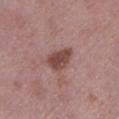This lesion was catalogued during total-body skin photography and was not selected for biopsy. A region of skin cropped from a whole-body photographic capture, roughly 15 mm wide. Measured at roughly 3.5 mm in maximum diameter. Imaged with white-light lighting. Located on the left lower leg. The subject is a female aged 63–67.